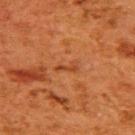The lesion was tiled from a total-body skin photograph and was not biopsied.
A region of skin cropped from a whole-body photographic capture, roughly 15 mm wide.
This is a cross-polarized tile.
A female subject aged approximately 50.
On the upper back.